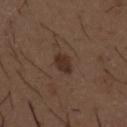Impression: Part of a total-body skin-imaging series; this lesion was reviewed on a skin check and was not flagged for biopsy. Clinical summary: The patient is a male about 50 years old. The lesion is located on the back. Cropped from a whole-body photographic skin survey; the tile spans about 15 mm. Automated tile analysis of the lesion measured an area of roughly 4.5 mm², an eccentricity of roughly 0.85, and two-axis asymmetry of about 0.25. The software also gave an average lesion color of about L≈30 a*≈16 b*≈24 (CIELAB), a lesion–skin lightness drop of about 9, and a normalized border contrast of about 8.5. Captured under white-light illumination. The lesion's longest dimension is about 3 mm.